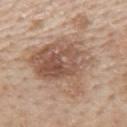– notes — catalogued during a skin exam; not biopsied
– size — ~9.5 mm (longest diameter)
– automated metrics — a mean CIELAB color near L≈57 a*≈17 b*≈28, roughly 11 lightness units darker than nearby skin, and a normalized lesion–skin contrast near 7; border irregularity of about 6 on a 0–10 scale and a peripheral color-asymmetry measure near 2.5; a detector confidence of about 100 out of 100 that the crop contains a lesion
– anatomic site — the back
– tile lighting — white-light
– subject — male, approximately 60 years of age
– image — ~15 mm crop, total-body skin-cancer survey